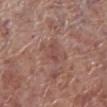Recorded during total-body skin imaging; not selected for excision or biopsy. The subject is a male about 70 years old. Cropped from a total-body skin-imaging series; the visible field is about 15 mm. About 2.5 mm across. Located on the right lower leg.